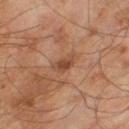The lesion was photographed on a routine skin check and not biopsied; there is no pathology result.
This image is a 15 mm lesion crop taken from a total-body photograph.
Longest diameter approximately 2.5 mm.
The total-body-photography lesion software estimated an average lesion color of about L≈45 a*≈21 b*≈31 (CIELAB) and about 10 CIELAB-L* units darker than the surrounding skin. The analysis additionally found a border-irregularity index near 3/10 and radial color variation of about 0.5.
A male subject approximately 65 years of age.
Imaged with cross-polarized lighting.